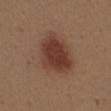Imaged during a routine full-body skin examination; the lesion was not biopsied and no histopathology is available. A female subject in their 40s. A lesion tile, about 15 mm wide, cut from a 3D total-body photograph. This is a white-light tile. The lesion is on the back.